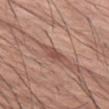Captured during whole-body skin photography for melanoma surveillance; the lesion was not biopsied.
A male subject, aged approximately 75.
The lesion is located on the left thigh.
A region of skin cropped from a whole-body photographic capture, roughly 15 mm wide.
Longest diameter approximately 3 mm.